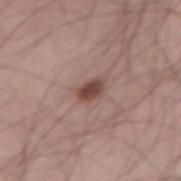biopsy status: total-body-photography surveillance lesion; no biopsy
diameter: ≈2.5 mm
patient: male, aged 48–52
automated metrics: a footprint of about 3.5 mm², an outline eccentricity of about 0.75 (0 = round, 1 = elongated), and two-axis asymmetry of about 0.15; an average lesion color of about L≈44 a*≈21 b*≈23 (CIELAB), about 13 CIELAB-L* units darker than the surrounding skin, and a normalized lesion–skin contrast near 10; border irregularity of about 1.5 on a 0–10 scale and peripheral color asymmetry of about 0.5; an automated nevus-likeness rating near 95 out of 100 and lesion-presence confidence of about 100/100
acquisition: ~15 mm tile from a whole-body skin photo
location: the right thigh